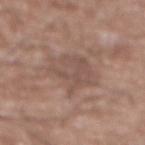image-analysis metrics=a footprint of about 16 mm², an eccentricity of roughly 0.5, and a symmetry-axis asymmetry near 0.25; a lesion color around L≈51 a*≈17 b*≈23 in CIELAB and a normalized lesion–skin contrast near 5; an automated nevus-likeness rating near 0 out of 100 and a lesion-detection confidence of about 95/100
subject=male, aged 73–77
imaging modality=total-body-photography crop, ~15 mm field of view
body site=the abdomen
lesion size=~5.5 mm (longest diameter)